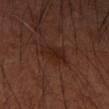The lesion was tiled from a total-body skin photograph and was not biopsied.
A region of skin cropped from a whole-body photographic capture, roughly 15 mm wide.
The tile uses cross-polarized illumination.
A male subject roughly 55 years of age.
Automated tile analysis of the lesion measured internal color variation of about 2.5 on a 0–10 scale and peripheral color asymmetry of about 1.
The lesion's longest dimension is about 4 mm.
The lesion is located on the left forearm.